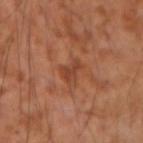Findings:
- biopsy status: total-body-photography surveillance lesion; no biopsy
- diameter: ≈2.5 mm
- site: the left forearm
- patient: male, aged 53 to 57
- image: ~15 mm crop, total-body skin-cancer survey